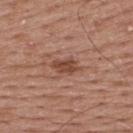| field | value |
|---|---|
| follow-up | total-body-photography surveillance lesion; no biopsy |
| patient | male, aged around 55 |
| anatomic site | the upper back |
| image | 15 mm crop, total-body photography |
| TBP lesion metrics | a lesion color around L≈46 a*≈23 b*≈28 in CIELAB, about 10 CIELAB-L* units darker than the surrounding skin, and a normalized border contrast of about 8 |
| lesion diameter | ~3.5 mm (longest diameter) |
| illumination | white-light illumination |